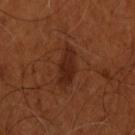Impression:
This lesion was catalogued during total-body skin photography and was not selected for biopsy.
Clinical summary:
Cropped from a whole-body photographic skin survey; the tile spans about 15 mm. From the head or neck. A male patient, aged approximately 55. This is a cross-polarized tile. An algorithmic analysis of the crop reported about 8 CIELAB-L* units darker than the surrounding skin. And it measured a nevus-likeness score of about 85/100 and a lesion-detection confidence of about 100/100.